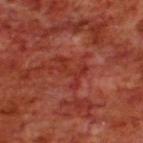Context:
Located on the upper back. A lesion tile, about 15 mm wide, cut from a 3D total-body photograph. Captured under cross-polarized illumination. The total-body-photography lesion software estimated border irregularity of about 8 on a 0–10 scale, a color-variation rating of about 1/10, and radial color variation of about 0.5. About 4 mm across. The patient is a male roughly 70 years of age.